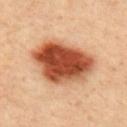Part of a total-body skin-imaging series; this lesion was reviewed on a skin check and was not flagged for biopsy. Imaged with cross-polarized lighting. A male subject roughly 40 years of age. A region of skin cropped from a whole-body photographic capture, roughly 15 mm wide. The lesion is located on the mid back. Approximately 7.5 mm at its widest. Automated image analysis of the tile measured a nevus-likeness score of about 100/100 and a lesion-detection confidence of about 100/100.